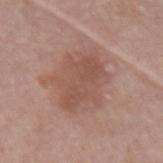Longest diameter approximately 6 mm. The tile uses white-light illumination. Cropped from a total-body skin-imaging series; the visible field is about 15 mm. A male patient about 55 years old. The lesion is located on the left forearm. An algorithmic analysis of the crop reported a footprint of about 18 mm², an outline eccentricity of about 0.65 (0 = round, 1 = elongated), and two-axis asymmetry of about 0.3. And it measured border irregularity of about 5.5 on a 0–10 scale, a within-lesion color-variation index near 2.5/10, and radial color variation of about 1. And it measured an automated nevus-likeness rating near 5 out of 100.